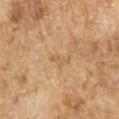Q: Is there a histopathology result?
A: total-body-photography surveillance lesion; no biopsy